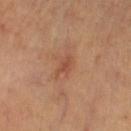Recorded during total-body skin imaging; not selected for excision or biopsy. The subject is aged approximately 60. Approximately 3 mm at its widest. An algorithmic analysis of the crop reported a footprint of about 2.5 mm² and an eccentricity of roughly 0.85. The analysis additionally found a border-irregularity index near 4.5/10 and a color-variation rating of about 0.5/10. It also reported a classifier nevus-likeness of about 0/100. The tile uses cross-polarized illumination. On the right thigh. This image is a 15 mm lesion crop taken from a total-body photograph.